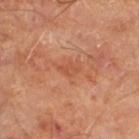Impression:
No biopsy was performed on this lesion — it was imaged during a full skin examination and was not determined to be concerning.
Acquisition and patient details:
Longest diameter approximately 2.5 mm. A 15 mm close-up tile from a total-body photography series done for melanoma screening. Automated image analysis of the tile measured a border-irregularity index near 2/10 and a color-variation rating of about 2/10. The analysis additionally found a classifier nevus-likeness of about 0/100 and a lesion-detection confidence of about 100/100. Located on the right thigh. A patient in their mid-60s.